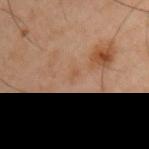Clinical impression: Captured during whole-body skin photography for melanoma surveillance; the lesion was not biopsied. Image and clinical context: Longest diameter approximately 2 mm. A male subject about 45 years old. On the left upper arm. Imaged with cross-polarized lighting. Cropped from a total-body skin-imaging series; the visible field is about 15 mm.